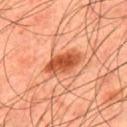  biopsy_status: not biopsied; imaged during a skin examination
  image:
    source: total-body photography crop
    field_of_view_mm: 15
  site: upper back
  lesion_size:
    long_diameter_mm_approx: 5.0
  patient:
    sex: male
    age_approx: 45
  lighting: cross-polarized